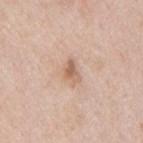Clinical impression:
The lesion was tiled from a total-body skin photograph and was not biopsied.
Context:
The subject is a male aged 58–62. Cropped from a total-body skin-imaging series; the visible field is about 15 mm. Located on the mid back. The tile uses white-light illumination.